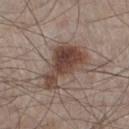| feature | finding |
|---|---|
| notes | imaged on a skin check; not biopsied |
| subject | male, aged 58 to 62 |
| site | the left lower leg |
| illumination | white-light illumination |
| image source | total-body-photography crop, ~15 mm field of view |
| diameter | ~6.5 mm (longest diameter) |